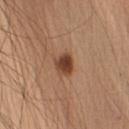| field | value |
|---|---|
| biopsy status | imaged on a skin check; not biopsied |
| location | the left upper arm |
| tile lighting | white-light illumination |
| diameter | ~2.5 mm (longest diameter) |
| image | 15 mm crop, total-body photography |
| subject | male, aged around 70 |